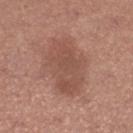notes — no biopsy performed (imaged during a skin exam)
acquisition — ~15 mm crop, total-body skin-cancer survey
anatomic site — the leg
patient — female, aged around 50
lesion diameter — ~6.5 mm (longest diameter)
illumination — white-light
automated metrics — a footprint of about 22 mm² and two-axis asymmetry of about 0.2; an average lesion color of about L≈50 a*≈22 b*≈27 (CIELAB), about 8 CIELAB-L* units darker than the surrounding skin, and a lesion-to-skin contrast of about 6 (normalized; higher = more distinct); a classifier nevus-likeness of about 15/100 and lesion-presence confidence of about 100/100